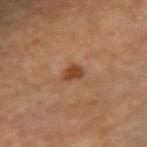Imaged during a routine full-body skin examination; the lesion was not biopsied and no histopathology is available.
A female subject aged 68 to 72.
A 15 mm close-up extracted from a 3D total-body photography capture.
An algorithmic analysis of the crop reported an average lesion color of about L≈42 a*≈21 b*≈32 (CIELAB) and a normalized lesion–skin contrast near 8.5. And it measured border irregularity of about 2.5 on a 0–10 scale, a within-lesion color-variation index near 1.5/10, and a peripheral color-asymmetry measure near 0.5.
The lesion is on the right forearm.
Captured under cross-polarized illumination.